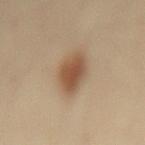Image and clinical context: A region of skin cropped from a whole-body photographic capture, roughly 15 mm wide. The patient is a female approximately 40 years of age. The tile uses cross-polarized illumination. The lesion is on the back.